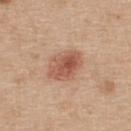workup = no biopsy performed (imaged during a skin exam); acquisition = 15 mm crop, total-body photography; lesion size = about 4.5 mm; patient = female, aged 43 to 47; lighting = white-light; site = the back.